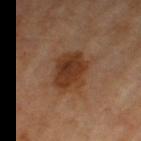workup: total-body-photography surveillance lesion; no biopsy
automated lesion analysis: a mean CIELAB color near L≈35 a*≈21 b*≈31 and a lesion-to-skin contrast of about 9.5 (normalized; higher = more distinct); a lesion-detection confidence of about 100/100
subject: roughly 70 years of age
lesion size: ~5 mm (longest diameter)
tile lighting: cross-polarized
image source: ~15 mm crop, total-body skin-cancer survey
body site: the left thigh